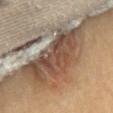biopsy status: total-body-photography surveillance lesion; no biopsy
image source: ~15 mm crop, total-body skin-cancer survey
tile lighting: cross-polarized illumination
subject: female, aged 63–67
automated metrics: a footprint of about 34 mm², a shape eccentricity near 0.9, and two-axis asymmetry of about 0.35; internal color variation of about 6 on a 0–10 scale and radial color variation of about 2
site: the chest